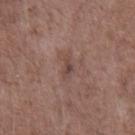This lesion was catalogued during total-body skin photography and was not selected for biopsy. This image is a 15 mm lesion crop taken from a total-body photograph. The patient is a male roughly 70 years of age. From the front of the torso. Longest diameter approximately 2.5 mm. This is a white-light tile.